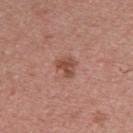Notes:
* biopsy status: total-body-photography surveillance lesion; no biopsy
* illumination: white-light illumination
* image: 15 mm crop, total-body photography
* anatomic site: the upper back
* patient: male, aged 38 to 42
* diameter: ~2.5 mm (longest diameter)